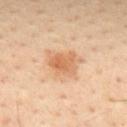lighting = cross-polarized illumination; lesion diameter = ~4 mm (longest diameter); subject = male, aged approximately 45; anatomic site = the mid back; imaging modality = ~15 mm tile from a whole-body skin photo.